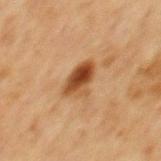Q: Who is the patient?
A: male, aged 63–67
Q: Where on the body is the lesion?
A: the mid back
Q: How was this image acquired?
A: total-body-photography crop, ~15 mm field of view
Q: What is the lesion's diameter?
A: about 4 mm
Q: How was the tile lit?
A: cross-polarized